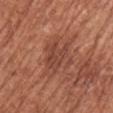{
  "biopsy_status": "not biopsied; imaged during a skin examination",
  "lighting": "white-light",
  "lesion_size": {
    "long_diameter_mm_approx": 5.0
  },
  "image": {
    "source": "total-body photography crop",
    "field_of_view_mm": 15
  },
  "site": "left upper arm",
  "patient": {
    "sex": "female",
    "age_approx": 75
  },
  "automated_metrics": {
    "cielab_L": 45,
    "cielab_a": 25,
    "cielab_b": 29,
    "vs_skin_contrast_norm": 6.0,
    "border_irregularity_0_10": 7.5,
    "color_variation_0_10": 3.0,
    "peripheral_color_asymmetry": 1.0,
    "nevus_likeness_0_100": 5,
    "lesion_detection_confidence_0_100": 95
  }
}